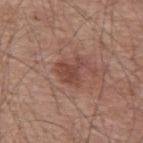{"biopsy_status": "not biopsied; imaged during a skin examination", "lighting": "white-light", "lesion_size": {"long_diameter_mm_approx": 3.5}, "patient": {"sex": "male", "age_approx": 55}, "image": {"source": "total-body photography crop", "field_of_view_mm": 15}, "site": "mid back"}